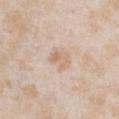Clinical impression: Part of a total-body skin-imaging series; this lesion was reviewed on a skin check and was not flagged for biopsy. Acquisition and patient details: An algorithmic analysis of the crop reported an area of roughly 4 mm² and an outline eccentricity of about 0.7 (0 = round, 1 = elongated). And it measured a border-irregularity index near 2.5/10 and internal color variation of about 4 on a 0–10 scale. It also reported an automated nevus-likeness rating near 5 out of 100. Imaged with white-light lighting. Located on the chest. A roughly 15 mm field-of-view crop from a total-body skin photograph. Approximately 2.5 mm at its widest. A female patient about 25 years old.